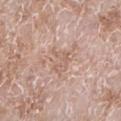The lesion was tiled from a total-body skin photograph and was not biopsied.
A 15 mm close-up extracted from a 3D total-body photography capture.
The lesion is on the left lower leg.
Imaged with white-light lighting.
The patient is a male about 60 years old.